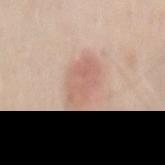The recorded lesion diameter is about 5 mm.
Automated image analysis of the tile measured a lesion area of about 12 mm² and an outline eccentricity of about 0.8 (0 = round, 1 = elongated). And it measured an average lesion color of about L≈63 a*≈21 b*≈27 (CIELAB) and a lesion-to-skin contrast of about 5.5 (normalized; higher = more distinct). It also reported a border-irregularity index near 2/10 and a peripheral color-asymmetry measure near 1. The software also gave a classifier nevus-likeness of about 50/100 and a detector confidence of about 100 out of 100 that the crop contains a lesion.
The subject is a male about 40 years old.
A 15 mm crop from a total-body photograph taken for skin-cancer surveillance.
The lesion is on the mid back.
This is a white-light tile.